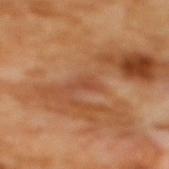Impression: The lesion was photographed on a routine skin check and not biopsied; there is no pathology result. Context: Located on the back. The patient is a female approximately 55 years of age. A 15 mm close-up tile from a total-body photography series done for melanoma screening.